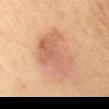Findings:
– workup: imaged on a skin check; not biopsied
– automated metrics: a footprint of about 19 mm² and two-axis asymmetry of about 0.45; a lesion color around L≈49 a*≈19 b*≈27 in CIELAB, a lesion–skin lightness drop of about 7, and a normalized border contrast of about 5.5; border irregularity of about 5 on a 0–10 scale, a within-lesion color-variation index near 3.5/10, and radial color variation of about 1
– size: ≈6 mm
– acquisition: total-body-photography crop, ~15 mm field of view
– subject: female, aged 58 to 62
– anatomic site: the chest